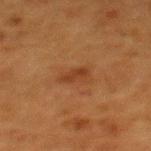Captured during whole-body skin photography for melanoma surveillance; the lesion was not biopsied. The lesion-visualizer software estimated a border-irregularity rating of about 2.5/10 and a peripheral color-asymmetry measure near 0.5. And it measured a lesion-detection confidence of about 100/100. Located on the upper back. This is a cross-polarized tile. Approximately 3 mm at its widest. A female patient, aged around 50. A 15 mm close-up extracted from a 3D total-body photography capture.